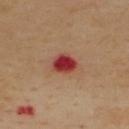lesion size: ≈3 mm | body site: the upper back | automated lesion analysis: a lesion color around L≈41 a*≈36 b*≈29 in CIELAB, about 16 CIELAB-L* units darker than the surrounding skin, and a lesion-to-skin contrast of about 12 (normalized; higher = more distinct); a border-irregularity rating of about 1.5/10, a within-lesion color-variation index near 4/10, and a peripheral color-asymmetry measure near 1; an automated nevus-likeness rating near 0 out of 100 and lesion-presence confidence of about 100/100 | subject: male, aged 53 to 57 | image source: total-body-photography crop, ~15 mm field of view.